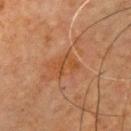Clinical impression: Part of a total-body skin-imaging series; this lesion was reviewed on a skin check and was not flagged for biopsy. Background: The lesion is located on the chest. A close-up tile cropped from a whole-body skin photograph, about 15 mm across. A male subject aged around 65.